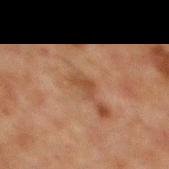Clinical summary: On the front of the torso. The patient is a male aged 63–67. Approximately 2.5 mm at its widest. Cropped from a whole-body photographic skin survey; the tile spans about 15 mm.